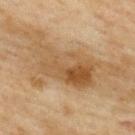Imaged during a routine full-body skin examination; the lesion was not biopsied and no histopathology is available.